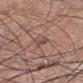| field | value |
|---|---|
| follow-up | imaged on a skin check; not biopsied |
| image source | 15 mm crop, total-body photography |
| subject | male, aged approximately 45 |
| TBP lesion metrics | an area of roughly 2.5 mm² and a shape-asymmetry score of about 0.25 (0 = symmetric); an average lesion color of about L≈48 a*≈22 b*≈23 (CIELAB) and about 8 CIELAB-L* units darker than the surrounding skin; lesion-presence confidence of about 60/100 |
| site | the left lower leg |